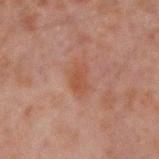- workup: imaged on a skin check; not biopsied